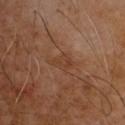Assessment: Part of a total-body skin-imaging series; this lesion was reviewed on a skin check and was not flagged for biopsy.